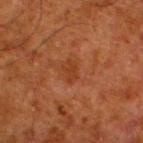| field | value |
|---|---|
| acquisition | ~15 mm tile from a whole-body skin photo |
| automated lesion analysis | a lesion area of about 2.5 mm², an outline eccentricity of about 0.85 (0 = round, 1 = elongated), and a symmetry-axis asymmetry near 0.45; a lesion-detection confidence of about 100/100 |
| lighting | cross-polarized |
| anatomic site | the left lower leg |
| patient | male, approximately 80 years of age |